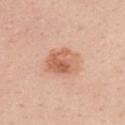<lesion>
<biopsy_status>not biopsied; imaged during a skin examination</biopsy_status>
<image>
  <source>total-body photography crop</source>
  <field_of_view_mm>15</field_of_view_mm>
</image>
<lesion_size>
  <long_diameter_mm_approx>4.5</long_diameter_mm_approx>
</lesion_size>
<lighting>white-light</lighting>
<patient>
  <sex>female</sex>
  <age_approx>40</age_approx>
</patient>
<automated_metrics>
  <area_mm2_approx>13.0</area_mm2_approx>
  <eccentricity>0.7</eccentricity>
  <cielab_L>63</cielab_L>
  <cielab_a>23</cielab_a>
  <cielab_b>33</cielab_b>
  <vs_skin_darker_L>10.0</vs_skin_darker_L>
</automated_metrics>
<site>mid back</site>
</lesion>